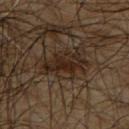Q: Was this lesion biopsied?
A: total-body-photography surveillance lesion; no biopsy
Q: What are the patient's age and sex?
A: male, in their mid-60s
Q: Illumination type?
A: cross-polarized
Q: How large is the lesion?
A: about 5 mm
Q: How was this image acquired?
A: total-body-photography crop, ~15 mm field of view
Q: Lesion location?
A: the upper back